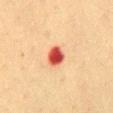A female patient, about 40 years old. Cropped from a total-body skin-imaging series; the visible field is about 15 mm. On the mid back. This is a cross-polarized tile. Longest diameter approximately 3 mm.